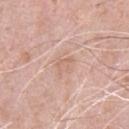The lesion was photographed on a routine skin check and not biopsied; there is no pathology result.